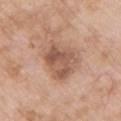Impression:
The lesion was photographed on a routine skin check and not biopsied; there is no pathology result.
Image and clinical context:
A female patient, aged around 75. The lesion's longest dimension is about 5 mm. The lesion is located on the chest. A 15 mm close-up extracted from a 3D total-body photography capture.